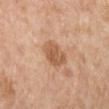Recorded during total-body skin imaging; not selected for excision or biopsy.
A close-up tile cropped from a whole-body skin photograph, about 15 mm across.
The lesion's longest dimension is about 3.5 mm.
The tile uses cross-polarized illumination.
On the right lower leg.
The patient is a female aged 53–57.